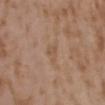Located on the upper back.
Approximately 2.5 mm at its widest.
A female subject aged 33–37.
A region of skin cropped from a whole-body photographic capture, roughly 15 mm wide.
Automated image analysis of the tile measured an average lesion color of about L≈53 a*≈17 b*≈30 (CIELAB), a lesion–skin lightness drop of about 5, and a normalized border contrast of about 4.5. And it measured a lesion-detection confidence of about 100/100.
Captured under white-light illumination.